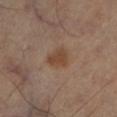Part of a total-body skin-imaging series; this lesion was reviewed on a skin check and was not flagged for biopsy.
Cropped from a whole-body photographic skin survey; the tile spans about 15 mm.
A female patient, in their 60s.
This is a cross-polarized tile.
The lesion is on the right leg.